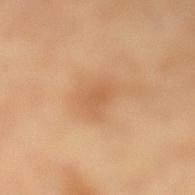Impression:
This lesion was catalogued during total-body skin photography and was not selected for biopsy.
Acquisition and patient details:
A female patient aged around 60. The lesion is located on the left lower leg. A lesion tile, about 15 mm wide, cut from a 3D total-body photograph. The tile uses cross-polarized illumination. The recorded lesion diameter is about 3.5 mm.